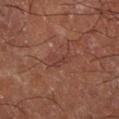| key | value |
|---|---|
| biopsy status | no biopsy performed (imaged during a skin exam) |
| imaging modality | ~15 mm tile from a whole-body skin photo |
| tile lighting | cross-polarized |
| patient | male, aged approximately 55 |
| site | the left upper arm |
| image-analysis metrics | an outline eccentricity of about 0.85 (0 = round, 1 = elongated) and a shape-asymmetry score of about 0.35 (0 = symmetric); border irregularity of about 3.5 on a 0–10 scale, internal color variation of about 2 on a 0–10 scale, and radial color variation of about 1 |